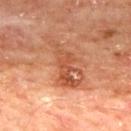| field | value |
|---|---|
| follow-up | catalogued during a skin exam; not biopsied |
| imaging modality | total-body-photography crop, ~15 mm field of view |
| lighting | cross-polarized |
| image-analysis metrics | an area of roughly 13 mm² and a shape eccentricity near 0.9; an automated nevus-likeness rating near 0 out of 100 |
| lesion diameter | about 7 mm |
| patient | male, aged around 65 |
| site | the mid back |